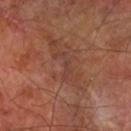Q: Was a biopsy performed?
A: catalogued during a skin exam; not biopsied
Q: What is the anatomic site?
A: the left forearm
Q: What kind of image is this?
A: ~15 mm tile from a whole-body skin photo
Q: How was the tile lit?
A: cross-polarized illumination
Q: What are the patient's age and sex?
A: male, aged around 70